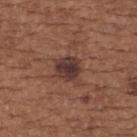location: the upper back | patient: female, roughly 65 years of age | lighting: white-light illumination | acquisition: total-body-photography crop, ~15 mm field of view | image-analysis metrics: an area of roughly 6 mm², an eccentricity of roughly 0.4, and two-axis asymmetry of about 0.15; a classifier nevus-likeness of about 45/100 and a lesion-detection confidence of about 100/100.